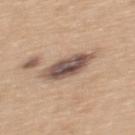Captured during whole-body skin photography for melanoma surveillance; the lesion was not biopsied.
A region of skin cropped from a whole-body photographic capture, roughly 15 mm wide.
The lesion is on the upper back.
Automated tile analysis of the lesion measured an average lesion color of about L≈52 a*≈16 b*≈24 (CIELAB) and a normalized border contrast of about 11.5. The analysis additionally found a border-irregularity rating of about 2/10, a color-variation rating of about 7/10, and radial color variation of about 2.5.
The patient is a female aged around 30.
This is a white-light tile.
The lesion's longest dimension is about 5 mm.